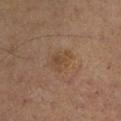This lesion was catalogued during total-body skin photography and was not selected for biopsy. The tile uses cross-polarized illumination. A 15 mm crop from a total-body photograph taken for skin-cancer surveillance. On the left lower leg. A male subject in their mid-60s.